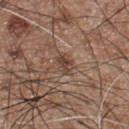| key | value |
|---|---|
| workup | total-body-photography surveillance lesion; no biopsy |
| TBP lesion metrics | an eccentricity of roughly 0.6 and a shape-asymmetry score of about 0.3 (0 = symmetric); a border-irregularity index near 2.5/10 and internal color variation of about 3 on a 0–10 scale |
| imaging modality | ~15 mm tile from a whole-body skin photo |
| diameter | ≈2.5 mm |
| body site | the chest |
| patient | male, in their mid-50s |
| tile lighting | white-light illumination |